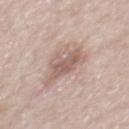{"biopsy_status": "not biopsied; imaged during a skin examination", "site": "chest", "lesion_size": {"long_diameter_mm_approx": 5.5}, "patient": {"sex": "male", "age_approx": 55}, "lighting": "white-light", "image": {"source": "total-body photography crop", "field_of_view_mm": 15}}